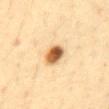follow-up=imaged on a skin check; not biopsied